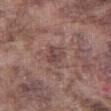The lesion's longest dimension is about 2.5 mm. A 15 mm crop from a total-body photograph taken for skin-cancer surveillance. Imaged with white-light lighting. The subject is a male aged 73 to 77. From the right thigh.